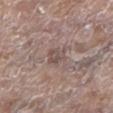| feature | finding |
|---|---|
| diameter | about 2.5 mm |
| site | the left lower leg |
| acquisition | ~15 mm tile from a whole-body skin photo |
| tile lighting | white-light illumination |
| subject | female, aged 83 or over |